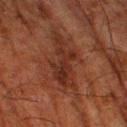Part of a total-body skin-imaging series; this lesion was reviewed on a skin check and was not flagged for biopsy. The tile uses cross-polarized illumination. A 15 mm close-up tile from a total-body photography series done for melanoma screening. Located on the right thigh. The total-body-photography lesion software estimated an automated nevus-likeness rating near 0 out of 100 and a lesion-detection confidence of about 95/100. Measured at roughly 6.5 mm in maximum diameter. The subject is a male about 80 years old.